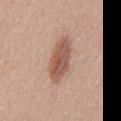Q: What is the anatomic site?
A: the chest
Q: What are the patient's age and sex?
A: male, aged 28 to 32
Q: What lighting was used for the tile?
A: white-light illumination
Q: How was this image acquired?
A: ~15 mm tile from a whole-body skin photo
Q: What is the lesion's diameter?
A: ~5 mm (longest diameter)